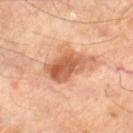notes: imaged on a skin check; not biopsied | image source: ~15 mm crop, total-body skin-cancer survey | body site: the leg | patient: male, aged approximately 70 | size: about 5 mm | tile lighting: cross-polarized | TBP lesion metrics: a lesion color around L≈58 a*≈26 b*≈36 in CIELAB, a lesion–skin lightness drop of about 14, and a lesion-to-skin contrast of about 9 (normalized; higher = more distinct); a within-lesion color-variation index near 5.5/10; a classifier nevus-likeness of about 65/100 and a detector confidence of about 100 out of 100 that the crop contains a lesion.